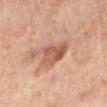<case>
  <biopsy_status>not biopsied; imaged during a skin examination</biopsy_status>
  <site>left lower leg</site>
  <patient>
    <sex>female</sex>
    <age_approx>55</age_approx>
  </patient>
  <automated_metrics>
    <nevus_likeness_0_100>0</nevus_likeness_0_100>
    <lesion_detection_confidence_0_100>100</lesion_detection_confidence_0_100>
  </automated_metrics>
  <image>
    <source>total-body photography crop</source>
    <field_of_view_mm>15</field_of_view_mm>
  </image>
</case>